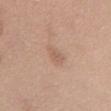follow-up: no biopsy performed (imaged during a skin exam) | subject: female, about 50 years old | tile lighting: white-light illumination | imaging modality: ~15 mm tile from a whole-body skin photo | anatomic site: the chest | lesion size: ≈3 mm.